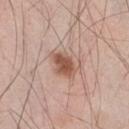This lesion was catalogued during total-body skin photography and was not selected for biopsy. A male subject, in their mid-40s. The total-body-photography lesion software estimated a border-irregularity rating of about 2/10, internal color variation of about 3.5 on a 0–10 scale, and peripheral color asymmetry of about 1. The lesion's longest dimension is about 3.5 mm. The lesion is located on the right thigh. A lesion tile, about 15 mm wide, cut from a 3D total-body photograph.